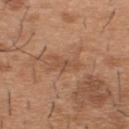Imaged with white-light lighting.
The lesion is located on the upper back.
A male patient aged around 40.
A close-up tile cropped from a whole-body skin photograph, about 15 mm across.
Automated image analysis of the tile measured a symmetry-axis asymmetry near 0.55. The analysis additionally found an average lesion color of about L≈50 a*≈21 b*≈32 (CIELAB), a lesion–skin lightness drop of about 6, and a lesion-to-skin contrast of about 4.5 (normalized; higher = more distinct).
About 3 mm across.